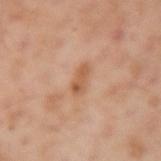notes: no biopsy performed (imaged during a skin exam) | acquisition: 15 mm crop, total-body photography | patient: male, aged approximately 55 | automated lesion analysis: an area of roughly 4 mm²; a classifier nevus-likeness of about 0/100 and a detector confidence of about 100 out of 100 that the crop contains a lesion | lesion diameter: ~3.5 mm (longest diameter) | location: the left upper arm.